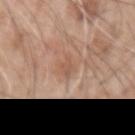The lesion was tiled from a total-body skin photograph and was not biopsied. Cropped from a whole-body photographic skin survey; the tile spans about 15 mm. Imaged with white-light lighting. Longest diameter approximately 2.5 mm. The lesion is located on the left upper arm. The subject is a male approximately 60 years of age.